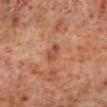Q: Was this lesion biopsied?
A: total-body-photography surveillance lesion; no biopsy
Q: Patient demographics?
A: male, aged 58 to 62
Q: Lesion location?
A: the left lower leg
Q: What kind of image is this?
A: total-body-photography crop, ~15 mm field of view
Q: What is the lesion's diameter?
A: about 2.5 mm